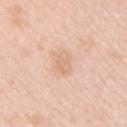Findings:
• notes — total-body-photography surveillance lesion; no biopsy
• imaging modality — ~15 mm crop, total-body skin-cancer survey
• body site — the chest
• patient — female, roughly 40 years of age
• TBP lesion metrics — a mean CIELAB color near L≈71 a*≈19 b*≈33, a lesion–skin lightness drop of about 7, and a normalized border contrast of about 4.5; a border-irregularity index near 2.5/10, a color-variation rating of about 2.5/10, and a peripheral color-asymmetry measure near 1